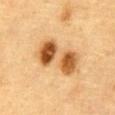No biopsy was performed on this lesion — it was imaged during a full skin examination and was not determined to be concerning.
A male subject aged 83 or over.
The lesion is located on the abdomen.
This is a cross-polarized tile.
The total-body-photography lesion software estimated an automated nevus-likeness rating near 100 out of 100 and a detector confidence of about 100 out of 100 that the crop contains a lesion.
A roughly 15 mm field-of-view crop from a total-body skin photograph.
The lesion's longest dimension is about 6.5 mm.